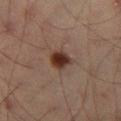Clinical impression: The lesion was photographed on a routine skin check and not biopsied; there is no pathology result. Image and clinical context: A roughly 15 mm field-of-view crop from a total-body skin photograph. The subject is a male aged 33 to 37. The recorded lesion diameter is about 3 mm. Automated tile analysis of the lesion measured a lesion color around L≈31 a*≈17 b*≈23 in CIELAB and roughly 12 lightness units darker than nearby skin. And it measured border irregularity of about 2 on a 0–10 scale, a within-lesion color-variation index near 4.5/10, and radial color variation of about 1.5. It also reported a nevus-likeness score of about 100/100. Located on the leg. Imaged with cross-polarized lighting.